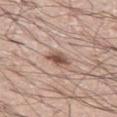Assessment:
Imaged during a routine full-body skin examination; the lesion was not biopsied and no histopathology is available.
Clinical summary:
The patient is a male roughly 70 years of age. A 15 mm close-up tile from a total-body photography series done for melanoma screening. From the right leg. The total-body-photography lesion software estimated an eccentricity of roughly 0.65 and a shape-asymmetry score of about 0.25 (0 = symmetric). And it measured about 13 CIELAB-L* units darker than the surrounding skin and a lesion-to-skin contrast of about 9 (normalized; higher = more distinct). The analysis additionally found border irregularity of about 2 on a 0–10 scale, a within-lesion color-variation index near 4/10, and a peripheral color-asymmetry measure near 1. Measured at roughly 2.5 mm in maximum diameter.